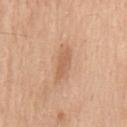follow-up = total-body-photography surveillance lesion; no biopsy
automated lesion analysis = an average lesion color of about L≈61 a*≈20 b*≈34 (CIELAB); border irregularity of about 3.5 on a 0–10 scale and internal color variation of about 1.5 on a 0–10 scale; a classifier nevus-likeness of about 30/100 and a detector confidence of about 100 out of 100 that the crop contains a lesion
site = the chest
image source = 15 mm crop, total-body photography
patient = male, roughly 60 years of age